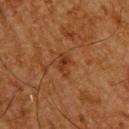notes: no biopsy performed (imaged during a skin exam) | location: the upper back | lighting: cross-polarized | image source: 15 mm crop, total-body photography | subject: male, in their mid- to late 60s.